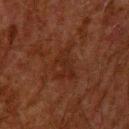Q: Was this lesion biopsied?
A: catalogued during a skin exam; not biopsied
Q: Patient demographics?
A: male, aged 58 to 62
Q: Illumination type?
A: cross-polarized illumination
Q: Lesion size?
A: about 5 mm
Q: Automated lesion metrics?
A: a lesion color around L≈20 a*≈18 b*≈23 in CIELAB, a lesion–skin lightness drop of about 4, and a normalized lesion–skin contrast near 5; an automated nevus-likeness rating near 0 out of 100 and a lesion-detection confidence of about 100/100
Q: What is the imaging modality?
A: ~15 mm crop, total-body skin-cancer survey
Q: Lesion location?
A: the upper back